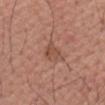Case summary:
– workup · imaged on a skin check; not biopsied
– patient · male, about 55 years old
– image source · 15 mm crop, total-body photography
– size · about 2.5 mm
– anatomic site · the head or neck
– lighting · white-light illumination